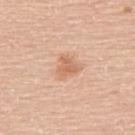Context:
Captured under white-light illumination. A 15 mm crop from a total-body photograph taken for skin-cancer surveillance. A male patient aged 58 to 62. Measured at roughly 2.5 mm in maximum diameter. On the upper back.